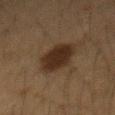Recorded during total-body skin imaging; not selected for excision or biopsy.
Longest diameter approximately 5 mm.
The lesion is located on the mid back.
This image is a 15 mm lesion crop taken from a total-body photograph.
A male subject, roughly 35 years of age.
The lesion-visualizer software estimated a border-irregularity index near 2.5/10, a within-lesion color-variation index near 2/10, and radial color variation of about 0.5. And it measured a nevus-likeness score of about 95/100 and a detector confidence of about 100 out of 100 that the crop contains a lesion.